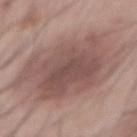Findings:
• follow-up · total-body-photography surveillance lesion; no biopsy
• automated metrics · two-axis asymmetry of about 0.15; an average lesion color of about L≈52 a*≈18 b*≈22 (CIELAB), roughly 10 lightness units darker than nearby skin, and a normalized lesion–skin contrast near 7; a border-irregularity index near 2.5/10, a within-lesion color-variation index near 4.5/10, and radial color variation of about 1.5; a classifier nevus-likeness of about 40/100
• body site · the abdomen
• lesion size · ~11 mm (longest diameter)
• illumination · white-light
• imaging modality · 15 mm crop, total-body photography
• patient · male, aged 53–57